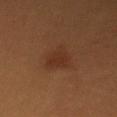{"biopsy_status": "not biopsied; imaged during a skin examination", "patient": {"sex": "female", "age_approx": 30}, "site": "chest", "lighting": "cross-polarized", "image": {"source": "total-body photography crop", "field_of_view_mm": 15}, "lesion_size": {"long_diameter_mm_approx": 4.5}, "automated_metrics": {"nevus_likeness_0_100": 90, "lesion_detection_confidence_0_100": 100}}